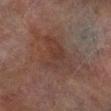subject: male, aged 73–77; diameter: ~6 mm (longest diameter); image: ~15 mm crop, total-body skin-cancer survey; lighting: cross-polarized; automated metrics: an average lesion color of about L≈31 a*≈15 b*≈20 (CIELAB) and about 5 CIELAB-L* units darker than the surrounding skin; site: the right lower leg.